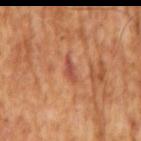- follow-up: imaged on a skin check; not biopsied
- image source: 15 mm crop, total-body photography
- patient: male, in their mid-60s